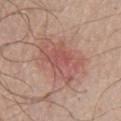follow-up: catalogued during a skin exam; not biopsied
imaging modality: total-body-photography crop, ~15 mm field of view
site: the chest
subject: male, roughly 70 years of age
lighting: white-light illumination
TBP lesion metrics: an area of roughly 18 mm², a shape eccentricity near 0.8, and a shape-asymmetry score of about 0.3 (0 = symmetric); radial color variation of about 1
diameter: ~6 mm (longest diameter)